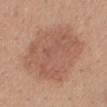– biopsy status — total-body-photography surveillance lesion; no biopsy
– patient — female, aged 28 to 32
– imaging modality — ~15 mm tile from a whole-body skin photo
– location — the mid back
– illumination — white-light